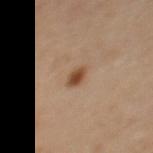<tbp_lesion>
  <biopsy_status>not biopsied; imaged during a skin examination</biopsy_status>
  <site>back</site>
  <lighting>cross-polarized</lighting>
  <image>
    <source>total-body photography crop</source>
    <field_of_view_mm>15</field_of_view_mm>
  </image>
  <patient>
    <sex>female</sex>
    <age_approx>40</age_approx>
  </patient>
</tbp_lesion>